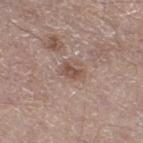Assessment: Captured during whole-body skin photography for melanoma surveillance; the lesion was not biopsied. Context: Cropped from a total-body skin-imaging series; the visible field is about 15 mm. Imaged with white-light lighting. About 2.5 mm across. The subject is a male in their mid- to late 60s. On the right thigh.